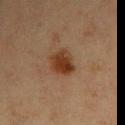follow-up=total-body-photography surveillance lesion; no biopsy | automated metrics=a shape eccentricity near 0.7 and two-axis asymmetry of about 0.2; a lesion color around L≈32 a*≈19 b*≈29 in CIELAB, a lesion–skin lightness drop of about 10, and a normalized lesion–skin contrast near 10.5; border irregularity of about 2 on a 0–10 scale and a color-variation rating of about 4/10; a classifier nevus-likeness of about 100/100 and a detector confidence of about 100 out of 100 that the crop contains a lesion | subject=female, in their mid- to late 40s | image source=~15 mm crop, total-body skin-cancer survey | tile lighting=cross-polarized | location=the right upper arm.